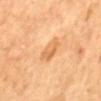The lesion was photographed on a routine skin check and not biopsied; there is no pathology result. The recorded lesion diameter is about 3.5 mm. From the back. Automated image analysis of the tile measured an area of roughly 6 mm², an eccentricity of roughly 0.85, and a shape-asymmetry score of about 0.25 (0 = symmetric). The analysis additionally found a border-irregularity rating of about 2.5/10 and a within-lesion color-variation index near 3.5/10. And it measured an automated nevus-likeness rating near 0 out of 100 and a lesion-detection confidence of about 100/100. A 15 mm close-up tile from a total-body photography series done for melanoma screening. A male subject in their mid-60s. This is a cross-polarized tile.